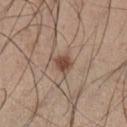No biopsy was performed on this lesion — it was imaged during a full skin examination and was not determined to be concerning. Located on the leg. A male subject approximately 35 years of age. The tile uses white-light illumination. The lesion-visualizer software estimated a border-irregularity rating of about 2/10 and a within-lesion color-variation index near 2/10. Cropped from a total-body skin-imaging series; the visible field is about 15 mm.